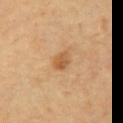Impression: No biopsy was performed on this lesion — it was imaged during a full skin examination and was not determined to be concerning. Acquisition and patient details: About 2.5 mm across. Captured under cross-polarized illumination. Automated tile analysis of the lesion measured an eccentricity of roughly 0.7 and two-axis asymmetry of about 0.15. It also reported a lesion color around L≈57 a*≈21 b*≈40 in CIELAB and a normalized border contrast of about 7. And it measured a border-irregularity index near 1.5/10, internal color variation of about 4 on a 0–10 scale, and radial color variation of about 1.5. And it measured a lesion-detection confidence of about 100/100. Located on the left upper arm. A subject aged 58 to 62. A 15 mm close-up extracted from a 3D total-body photography capture.